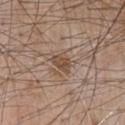The lesion was tiled from a total-body skin photograph and was not biopsied. About 3 mm across. From the chest. Captured under white-light illumination. A roughly 15 mm field-of-view crop from a total-body skin photograph. A male patient, aged 58–62.